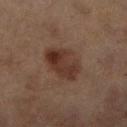No biopsy was performed on this lesion — it was imaged during a full skin examination and was not determined to be concerning. Cropped from a total-body skin-imaging series; the visible field is about 15 mm. About 5.5 mm across. The lesion is on the right lower leg. Automated image analysis of the tile measured an area of roughly 13 mm², a shape eccentricity near 0.8, and a symmetry-axis asymmetry near 0.15. It also reported border irregularity of about 2 on a 0–10 scale, internal color variation of about 5 on a 0–10 scale, and a peripheral color-asymmetry measure near 2. And it measured a classifier nevus-likeness of about 85/100. The patient is a female in their 60s.